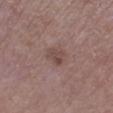{"biopsy_status": "not biopsied; imaged during a skin examination", "patient": {"sex": "male", "age_approx": 75}, "image": {"source": "total-body photography crop", "field_of_view_mm": 15}, "site": "right lower leg"}